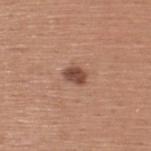{"biopsy_status": "not biopsied; imaged during a skin examination", "patient": {"sex": "male", "age_approx": 45}, "image": {"source": "total-body photography crop", "field_of_view_mm": 15}, "lesion_size": {"long_diameter_mm_approx": 2.5}, "site": "upper back"}